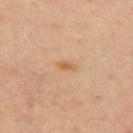patient:
  sex: male
  age_approx: 55
lighting: cross-polarized
lesion_size:
  long_diameter_mm_approx: 2.0
automated_metrics:
  area_mm2_approx: 2.0
  eccentricity: 0.85
  shape_asymmetry: 0.4
  color_variation_0_10: 0.5
  peripheral_color_asymmetry: 0.0
  nevus_likeness_0_100: 15
  lesion_detection_confidence_0_100: 100
site: right upper arm
image:
  source: total-body photography crop
  field_of_view_mm: 15